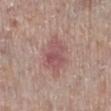imaging modality=~15 mm crop, total-body skin-cancer survey | body site=the left lower leg | patient=female, in their mid-60s | lesion size=~5 mm (longest diameter).